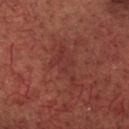follow-up = no biopsy performed (imaged during a skin exam) | automated metrics = an average lesion color of about L≈30 a*≈25 b*≈22 (CIELAB) and a normalized border contrast of about 4.5; a border-irregularity index near 9/10, internal color variation of about 2 on a 0–10 scale, and peripheral color asymmetry of about 0.5 | illumination = cross-polarized illumination | anatomic site = the head or neck | subject = male, approximately 60 years of age | lesion diameter = about 5.5 mm | image source = 15 mm crop, total-body photography.